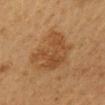The patient is a male aged around 60.
A roughly 15 mm field-of-view crop from a total-body skin photograph.
From the back.